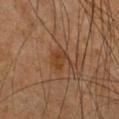follow-up: no biopsy performed (imaged during a skin exam)
illumination: cross-polarized illumination
TBP lesion metrics: an automated nevus-likeness rating near 0 out of 100
anatomic site: the chest
image: ~15 mm tile from a whole-body skin photo
size: ≈2.5 mm
subject: male, about 50 years old